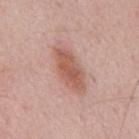Imaged during a routine full-body skin examination; the lesion was not biopsied and no histopathology is available. The lesion is on the mid back. A male subject aged 53 to 57. About 6 mm across. A lesion tile, about 15 mm wide, cut from a 3D total-body photograph.